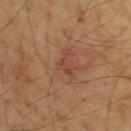Clinical summary: A male subject aged approximately 55. A 15 mm close-up extracted from a 3D total-body photography capture. Captured under cross-polarized illumination. Located on the upper back.